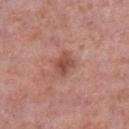<record>
<biopsy_status>not biopsied; imaged during a skin examination</biopsy_status>
<patient>
  <sex>male</sex>
  <age_approx>55</age_approx>
</patient>
<image>
  <source>total-body photography crop</source>
  <field_of_view_mm>15</field_of_view_mm>
</image>
<site>left lower leg</site>
</record>